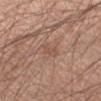Impression: Part of a total-body skin-imaging series; this lesion was reviewed on a skin check and was not flagged for biopsy. Acquisition and patient details: This is a white-light tile. An algorithmic analysis of the crop reported a footprint of about 2.5 mm², an eccentricity of roughly 0.9, and a shape-asymmetry score of about 0.35 (0 = symmetric). The recorded lesion diameter is about 2.5 mm. Located on the left forearm. A male patient, aged 33 to 37. This image is a 15 mm lesion crop taken from a total-body photograph.